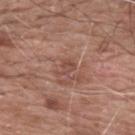patient:
  sex: male
  age_approx: 60
site: back
image:
  source: total-body photography crop
  field_of_view_mm: 15
lesion_size:
  long_diameter_mm_approx: 2.5
lighting: white-light